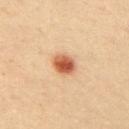Part of a total-body skin-imaging series; this lesion was reviewed on a skin check and was not flagged for biopsy.
Measured at roughly 2.5 mm in maximum diameter.
The subject is a male approximately 40 years of age.
Cropped from a whole-body photographic skin survey; the tile spans about 15 mm.
The tile uses cross-polarized illumination.
Automated image analysis of the tile measured an area of roughly 5.5 mm², a shape eccentricity near 0.45, and two-axis asymmetry of about 0.2. And it measured a mean CIELAB color near L≈52 a*≈25 b*≈34. The software also gave a color-variation rating of about 5.5/10.
The lesion is on the right upper arm.